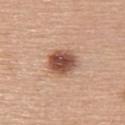Notes:
- notes · total-body-photography surveillance lesion; no biopsy
- acquisition · ~15 mm tile from a whole-body skin photo
- location · the upper back
- lesion size · ≈4 mm
- image-analysis metrics · roughly 16 lightness units darker than nearby skin and a normalized border contrast of about 11; a border-irregularity rating of about 1.5/10 and a color-variation rating of about 5/10
- tile lighting · white-light
- patient · female, approximately 65 years of age